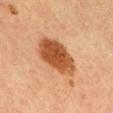Q: Was a biopsy performed?
A: no biopsy performed (imaged during a skin exam)
Q: What are the patient's age and sex?
A: male, aged 33–37
Q: Where on the body is the lesion?
A: the chest
Q: What did automated image analysis measure?
A: a border-irregularity rating of about 1.5/10, a color-variation rating of about 4/10, and a peripheral color-asymmetry measure near 1.5; a classifier nevus-likeness of about 100/100 and lesion-presence confidence of about 100/100
Q: What lighting was used for the tile?
A: cross-polarized illumination
Q: What is the imaging modality?
A: ~15 mm crop, total-body skin-cancer survey